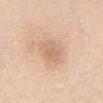The lesion was photographed on a routine skin check and not biopsied; there is no pathology result. About 4 mm across. The subject is a female aged 53 to 57. A region of skin cropped from a whole-body photographic capture, roughly 15 mm wide. Imaged with white-light lighting. From the abdomen.